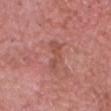{
  "lighting": "white-light",
  "image": {
    "source": "total-body photography crop",
    "field_of_view_mm": 15
  },
  "lesion_size": {
    "long_diameter_mm_approx": 4.5
  },
  "patient": {
    "sex": "male",
    "age_approx": 65
  },
  "site": "head or neck",
  "automated_metrics": {
    "area_mm2_approx": 6.5,
    "color_variation_0_10": 1.5,
    "peripheral_color_asymmetry": 0.5,
    "nevus_likeness_0_100": 0,
    "lesion_detection_confidence_0_100": 100
  }
}